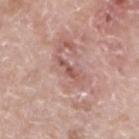This lesion was catalogued during total-body skin photography and was not selected for biopsy.
Located on the right forearm.
A 15 mm close-up tile from a total-body photography series done for melanoma screening.
A male subject, about 50 years old.